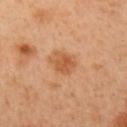| feature | finding |
|---|---|
| notes | catalogued during a skin exam; not biopsied |
| lesion diameter | about 3.5 mm |
| subject | female, approximately 40 years of age |
| location | the left upper arm |
| illumination | cross-polarized illumination |
| image source | 15 mm crop, total-body photography |